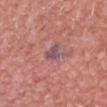No biopsy was performed on this lesion — it was imaged during a full skin examination and was not determined to be concerning. Imaged with white-light lighting. A roughly 15 mm field-of-view crop from a total-body skin photograph. Automated image analysis of the tile measured a mean CIELAB color near L≈50 a*≈21 b*≈15 and roughly 9 lightness units darker than nearby skin. It also reported a nevus-likeness score of about 0/100 and a lesion-detection confidence of about 95/100. The lesion is located on the head or neck. The subject is a male aged around 60.